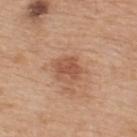Captured during whole-body skin photography for melanoma surveillance; the lesion was not biopsied.
From the upper back.
Captured under white-light illumination.
Approximately 3 mm at its widest.
A male subject, aged 63 to 67.
This image is a 15 mm lesion crop taken from a total-body photograph.